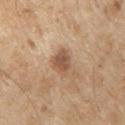<record>
<biopsy_status>not biopsied; imaged during a skin examination</biopsy_status>
</record>